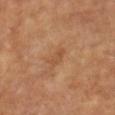Findings:
• biopsy status · imaged on a skin check; not biopsied
• subject · female, about 75 years old
• image source · ~15 mm tile from a whole-body skin photo
• illumination · cross-polarized
• anatomic site · the left upper arm
• diameter · ~2.5 mm (longest diameter)
• image-analysis metrics · two-axis asymmetry of about 0.25; an average lesion color of about L≈51 a*≈22 b*≈36 (CIELAB), a lesion–skin lightness drop of about 6, and a normalized lesion–skin contrast near 5; a border-irregularity rating of about 2.5/10, a within-lesion color-variation index near 1.5/10, and a peripheral color-asymmetry measure near 0.5